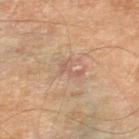This lesion was catalogued during total-body skin photography and was not selected for biopsy. A male patient, roughly 70 years of age. A 15 mm close-up extracted from a 3D total-body photography capture. The total-body-photography lesion software estimated an average lesion color of about L≈57 a*≈19 b*≈28 (CIELAB), roughly 7 lightness units darker than nearby skin, and a lesion-to-skin contrast of about 5 (normalized; higher = more distinct). The analysis additionally found a border-irregularity index near 6/10, internal color variation of about 0.5 on a 0–10 scale, and a peripheral color-asymmetry measure near 0. The tile uses cross-polarized illumination. The lesion is located on the leg.